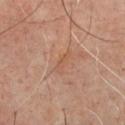follow-up: imaged on a skin check; not biopsied
image-analysis metrics: a footprint of about 3 mm², an outline eccentricity of about 0.95 (0 = round, 1 = elongated), and a symmetry-axis asymmetry near 0.35; a mean CIELAB color near L≈53 a*≈21 b*≈31, roughly 5 lightness units darker than nearby skin, and a normalized border contrast of about 5; a border-irregularity index near 4/10 and a within-lesion color-variation index near 0/10; an automated nevus-likeness rating near 0 out of 100 and a detector confidence of about 85 out of 100 that the crop contains a lesion
patient: in their mid-60s
tile lighting: cross-polarized
diameter: ~3 mm (longest diameter)
location: the chest
imaging modality: ~15 mm tile from a whole-body skin photo